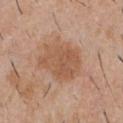Captured during whole-body skin photography for melanoma surveillance; the lesion was not biopsied.
A 15 mm close-up tile from a total-body photography series done for melanoma screening.
The lesion is on the chest.
The subject is a male aged 28–32.